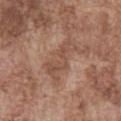On the abdomen.
Cropped from a total-body skin-imaging series; the visible field is about 15 mm.
Automated tile analysis of the lesion measured a within-lesion color-variation index near 2/10.
The lesion's longest dimension is about 5.5 mm.
A male patient in their mid-70s.
This is a white-light tile.